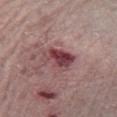Q: Patient demographics?
A: male, roughly 80 years of age
Q: How was the tile lit?
A: white-light illumination
Q: Where on the body is the lesion?
A: the left forearm
Q: What is the imaging modality?
A: ~15 mm crop, total-body skin-cancer survey
Q: How large is the lesion?
A: ~4 mm (longest diameter)